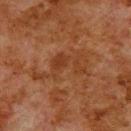Impression: Recorded during total-body skin imaging; not selected for excision or biopsy. Context: Captured under cross-polarized illumination. A lesion tile, about 15 mm wide, cut from a 3D total-body photograph. The lesion is located on the upper back. The recorded lesion diameter is about 6.5 mm. The patient is a male roughly 80 years of age.